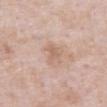Findings:
- patient — male, about 55 years old
- acquisition — ~15 mm crop, total-body skin-cancer survey
- site — the abdomen
- automated metrics — about 7 CIELAB-L* units darker than the surrounding skin and a normalized border contrast of about 5; an automated nevus-likeness rating near 0 out of 100 and lesion-presence confidence of about 100/100